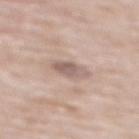Captured during whole-body skin photography for melanoma surveillance; the lesion was not biopsied.
The total-body-photography lesion software estimated a lesion area of about 5.5 mm² and an outline eccentricity of about 0.7 (0 = round, 1 = elongated). It also reported a mean CIELAB color near L≈59 a*≈16 b*≈22, a lesion–skin lightness drop of about 10, and a normalized border contrast of about 7. And it measured border irregularity of about 1.5 on a 0–10 scale, a within-lesion color-variation index near 4/10, and radial color variation of about 1.5.
A male subject approximately 80 years of age.
From the mid back.
A 15 mm crop from a total-body photograph taken for skin-cancer surveillance.
The lesion's longest dimension is about 3 mm.